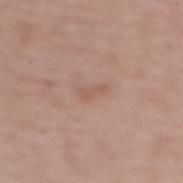Impression:
The lesion was photographed on a routine skin check and not biopsied; there is no pathology result.
Context:
The lesion is located on the upper back. A female patient in their mid- to late 60s. Cropped from a total-body skin-imaging series; the visible field is about 15 mm. Captured under white-light illumination. The lesion-visualizer software estimated an area of roughly 2.5 mm², an eccentricity of roughly 0.9, and a symmetry-axis asymmetry near 0.4. The analysis additionally found a border-irregularity rating of about 4.5/10, internal color variation of about 0 on a 0–10 scale, and a peripheral color-asymmetry measure near 0. The software also gave an automated nevus-likeness rating near 0 out of 100 and a lesion-detection confidence of about 100/100. The recorded lesion diameter is about 3 mm.